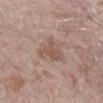* notes: imaged on a skin check; not biopsied
* imaging modality: ~15 mm crop, total-body skin-cancer survey
* anatomic site: the leg
* patient: male, about 45 years old
* illumination: white-light illumination
* image-analysis metrics: a lesion area of about 9.5 mm², an eccentricity of roughly 0.65, and two-axis asymmetry of about 0.4; a nevus-likeness score of about 0/100 and a lesion-detection confidence of about 100/100
* size: ~4.5 mm (longest diameter)